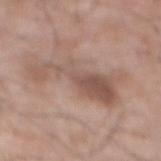Notes:
* notes: imaged on a skin check; not biopsied
* patient: male, aged around 70
* illumination: white-light
* size: ≈8.5 mm
* acquisition: ~15 mm crop, total-body skin-cancer survey
* body site: the abdomen
* image-analysis metrics: a lesion area of about 19 mm², an eccentricity of roughly 0.95, and a symmetry-axis asymmetry near 0.6; a border-irregularity rating of about 9.5/10 and a within-lesion color-variation index near 4.5/10